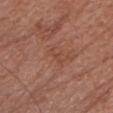  biopsy_status: not biopsied; imaged during a skin examination
  patient:
    sex: female
    age_approx: 75
  image:
    source: total-body photography crop
    field_of_view_mm: 15
  lighting: white-light
  site: head or neck